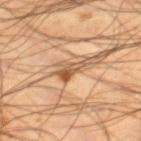notes: catalogued during a skin exam; not biopsied
anatomic site: the right lower leg
automated metrics: an average lesion color of about L≈54 a*≈21 b*≈37 (CIELAB) and a lesion-to-skin contrast of about 9 (normalized; higher = more distinct); a border-irregularity index near 4.5/10 and internal color variation of about 5.5 on a 0–10 scale; an automated nevus-likeness rating near 30 out of 100 and a detector confidence of about 95 out of 100 that the crop contains a lesion
size: ≈3 mm
image source: ~15 mm crop, total-body skin-cancer survey
subject: male, roughly 45 years of age
lighting: cross-polarized illumination